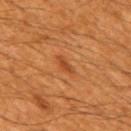<lesion>
<biopsy_status>not biopsied; imaged during a skin examination</biopsy_status>
<patient>
  <sex>male</sex>
  <age_approx>60</age_approx>
</patient>
<site>mid back</site>
<image>
  <source>total-body photography crop</source>
  <field_of_view_mm>15</field_of_view_mm>
</image>
</lesion>